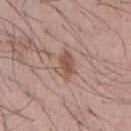Part of a total-body skin-imaging series; this lesion was reviewed on a skin check and was not flagged for biopsy.
Captured under white-light illumination.
Measured at roughly 3.5 mm in maximum diameter.
A male subject aged 43 to 47.
A roughly 15 mm field-of-view crop from a total-body skin photograph.
The total-body-photography lesion software estimated a footprint of about 6 mm² and two-axis asymmetry of about 0.25. And it measured a classifier nevus-likeness of about 60/100 and a detector confidence of about 100 out of 100 that the crop contains a lesion.
On the chest.